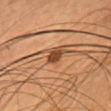subject: female, aged 33–37; location: the head or neck; illumination: cross-polarized; image: ~15 mm crop, total-body skin-cancer survey.